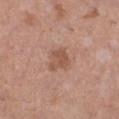{"biopsy_status": "not biopsied; imaged during a skin examination", "image": {"source": "total-body photography crop", "field_of_view_mm": 15}, "patient": {"sex": "female", "age_approx": 30}, "site": "leg", "automated_metrics": {"area_mm2_approx": 6.0, "shape_asymmetry": 0.3, "cielab_L": 53, "cielab_a": 22, "cielab_b": 29, "vs_skin_contrast_norm": 6.5, "border_irregularity_0_10": 3.0, "color_variation_0_10": 2.5, "peripheral_color_asymmetry": 1.0, "nevus_likeness_0_100": 10}, "lesion_size": {"long_diameter_mm_approx": 3.0}}